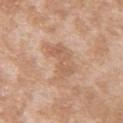biopsy status=total-body-photography surveillance lesion; no biopsy
imaging modality=~15 mm tile from a whole-body skin photo
body site=the back
subject=female, in their mid-20s